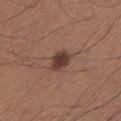workup: catalogued during a skin exam; not biopsied
subject: male, in their mid- to late 60s
location: the left lower leg
imaging modality: total-body-photography crop, ~15 mm field of view
illumination: white-light
size: ≈3 mm